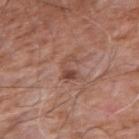Q: What is the anatomic site?
A: the right upper arm
Q: Patient demographics?
A: male, roughly 60 years of age
Q: What is the lesion's diameter?
A: ≈3.5 mm
Q: How was this image acquired?
A: 15 mm crop, total-body photography